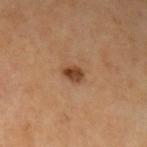- workup: total-body-photography surveillance lesion; no biopsy
- acquisition: total-body-photography crop, ~15 mm field of view
- patient: aged 63–67
- site: the left upper arm